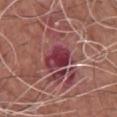Q: Is there a histopathology result?
A: catalogued during a skin exam; not biopsied
Q: What is the lesion's diameter?
A: ≈4 mm
Q: What is the anatomic site?
A: the chest
Q: What are the patient's age and sex?
A: male, aged 73–77
Q: How was this image acquired?
A: total-body-photography crop, ~15 mm field of view
Q: Automated lesion metrics?
A: a classifier nevus-likeness of about 0/100 and a lesion-detection confidence of about 90/100